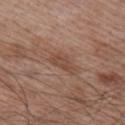Imaged during a routine full-body skin examination; the lesion was not biopsied and no histopathology is available. This is a white-light tile. The lesion is located on the right upper arm. A 15 mm close-up extracted from a 3D total-body photography capture. The subject is a male approximately 65 years of age. Approximately 3 mm at its widest.